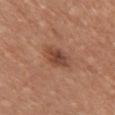Assessment:
The lesion was photographed on a routine skin check and not biopsied; there is no pathology result.
Context:
Approximately 3.5 mm at its widest. On the chest. The total-body-photography lesion software estimated a lesion area of about 6 mm² and two-axis asymmetry of about 0.25. It also reported an average lesion color of about L≈45 a*≈22 b*≈29 (CIELAB), a lesion–skin lightness drop of about 11, and a normalized border contrast of about 8. This image is a 15 mm lesion crop taken from a total-body photograph. A female subject aged approximately 55.